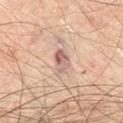Impression:
Part of a total-body skin-imaging series; this lesion was reviewed on a skin check and was not flagged for biopsy.
Image and clinical context:
From the abdomen. Automated tile analysis of the lesion measured a border-irregularity rating of about 2/10. The tile uses cross-polarized illumination. A male patient, about 65 years old. About 3.5 mm across. This image is a 15 mm lesion crop taken from a total-body photograph.